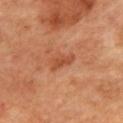<case>
<biopsy_status>not biopsied; imaged during a skin examination</biopsy_status>
<site>mid back</site>
<automated_metrics>
  <nevus_likeness_0_100>0</nevus_likeness_0_100>
  <lesion_detection_confidence_0_100>100</lesion_detection_confidence_0_100>
</automated_metrics>
<lighting>cross-polarized</lighting>
<image>
  <source>total-body photography crop</source>
  <field_of_view_mm>15</field_of_view_mm>
</image>
<patient>
  <age_approx>65</age_approx>
</patient>
</case>